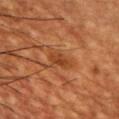{"biopsy_status": "not biopsied; imaged during a skin examination", "site": "upper back", "image": {"source": "total-body photography crop", "field_of_view_mm": 15}, "lesion_size": {"long_diameter_mm_approx": 2.5}, "patient": {"sex": "male", "age_approx": 55}}